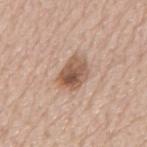Findings:
- follow-up: imaged on a skin check; not biopsied
- image: total-body-photography crop, ~15 mm field of view
- size: ~4 mm (longest diameter)
- image-analysis metrics: border irregularity of about 2.5 on a 0–10 scale, a color-variation rating of about 7.5/10, and peripheral color asymmetry of about 3; a classifier nevus-likeness of about 45/100
- subject: male, approximately 75 years of age
- anatomic site: the mid back
- illumination: white-light